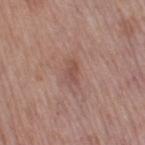Recorded during total-body skin imaging; not selected for excision or biopsy. Imaged with white-light lighting. The subject is a female aged approximately 50. The lesion-visualizer software estimated a footprint of about 3 mm² and a symmetry-axis asymmetry near 0.4. And it measured a mean CIELAB color near L≈50 a*≈21 b*≈25, a lesion–skin lightness drop of about 8, and a normalized lesion–skin contrast near 6. The analysis additionally found internal color variation of about 0.5 on a 0–10 scale and a peripheral color-asymmetry measure near 0. The lesion's longest dimension is about 2.5 mm. The lesion is on the right thigh. This image is a 15 mm lesion crop taken from a total-body photograph.